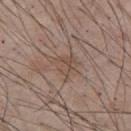biopsy status: imaged on a skin check; not biopsied